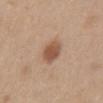  automated_metrics:
    area_mm2_approx: 7.0
    eccentricity: 0.75
    shape_asymmetry: 0.15
    vs_skin_darker_L: 12.0
    vs_skin_contrast_norm: 8.5
    border_irregularity_0_10: 1.5
    color_variation_0_10: 3.0
    peripheral_color_asymmetry: 1.0
    lesion_detection_confidence_0_100: 100
  lighting: white-light
  patient:
    sex: female
    age_approx: 45
  site: chest
  image:
    source: total-body photography crop
    field_of_view_mm: 15